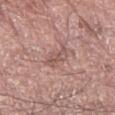| key | value |
|---|---|
| notes | total-body-photography surveillance lesion; no biopsy |
| body site | the right forearm |
| illumination | white-light |
| imaging modality | total-body-photography crop, ~15 mm field of view |
| patient | male, aged approximately 75 |